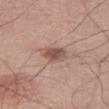This lesion was catalogued during total-body skin photography and was not selected for biopsy. The lesion is located on the right thigh. About 3.5 mm across. The tile uses white-light illumination. The subject is a male roughly 70 years of age. A region of skin cropped from a whole-body photographic capture, roughly 15 mm wide.